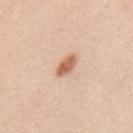biopsy status: no biopsy performed (imaged during a skin exam)
imaging modality: ~15 mm tile from a whole-body skin photo
tile lighting: white-light
patient: male, aged around 35
site: the mid back
size: about 3 mm